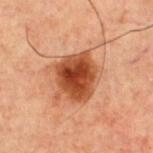No biopsy was performed on this lesion — it was imaged during a full skin examination and was not determined to be concerning. Automated tile analysis of the lesion measured a lesion color around L≈36 a*≈24 b*≈30 in CIELAB, about 14 CIELAB-L* units darker than the surrounding skin, and a normalized border contrast of about 12. And it measured a border-irregularity rating of about 2.5/10, internal color variation of about 5.5 on a 0–10 scale, and radial color variation of about 1.5. Captured under cross-polarized illumination. Measured at roughly 5 mm in maximum diameter. A male subject, approximately 60 years of age. A roughly 15 mm field-of-view crop from a total-body skin photograph. On the chest.